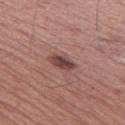Recorded during total-body skin imaging; not selected for excision or biopsy. An algorithmic analysis of the crop reported a footprint of about 5 mm², an outline eccentricity of about 0.85 (0 = round, 1 = elongated), and a symmetry-axis asymmetry near 0.25. The analysis additionally found a nevus-likeness score of about 75/100 and a lesion-detection confidence of about 100/100. From the left thigh. A roughly 15 mm field-of-view crop from a total-body skin photograph. The subject is a male aged approximately 50. This is a white-light tile. The recorded lesion diameter is about 3.5 mm.